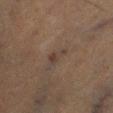No biopsy was performed on this lesion — it was imaged during a full skin examination and was not determined to be concerning.